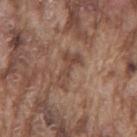Impression:
Part of a total-body skin-imaging series; this lesion was reviewed on a skin check and was not flagged for biopsy.
Context:
A 15 mm crop from a total-body photograph taken for skin-cancer surveillance. A male subject, approximately 75 years of age. From the mid back.